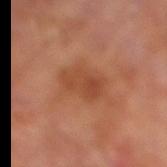Clinical impression: Part of a total-body skin-imaging series; this lesion was reviewed on a skin check and was not flagged for biopsy. Image and clinical context: The tile uses cross-polarized illumination. A 15 mm crop from a total-body photograph taken for skin-cancer surveillance. The subject is a male approximately 70 years of age. The lesion is on the leg. The lesion-visualizer software estimated a mean CIELAB color near L≈45 a*≈26 b*≈34, roughly 8 lightness units darker than nearby skin, and a lesion-to-skin contrast of about 6.5 (normalized; higher = more distinct). It also reported border irregularity of about 3.5 on a 0–10 scale, internal color variation of about 3.5 on a 0–10 scale, and a peripheral color-asymmetry measure near 1. The software also gave a classifier nevus-likeness of about 0/100 and a lesion-detection confidence of about 100/100.